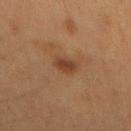Imaged during a routine full-body skin examination; the lesion was not biopsied and no histopathology is available.
A roughly 15 mm field-of-view crop from a total-body skin photograph.
The lesion is located on the mid back.
A male subject aged 63–67.